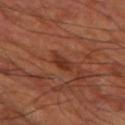The lesion was tiled from a total-body skin photograph and was not biopsied.
Imaged with cross-polarized lighting.
A patient about 65 years old.
Automated tile analysis of the lesion measured a lesion color around L≈33 a*≈24 b*≈30 in CIELAB, a lesion–skin lightness drop of about 8, and a normalized border contrast of about 7.5.
The recorded lesion diameter is about 3 mm.
Located on the leg.
This image is a 15 mm lesion crop taken from a total-body photograph.